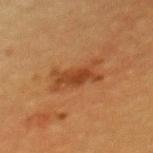Q: Lesion size?
A: ≈5.5 mm
Q: Automated lesion metrics?
A: a border-irregularity rating of about 5/10 and internal color variation of about 3 on a 0–10 scale; an automated nevus-likeness rating near 35 out of 100 and a detector confidence of about 100 out of 100 that the crop contains a lesion
Q: How was this image acquired?
A: ~15 mm tile from a whole-body skin photo
Q: Who is the patient?
A: female, aged 53 to 57
Q: Where on the body is the lesion?
A: the mid back